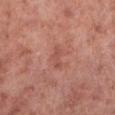biopsy status=imaged on a skin check; not biopsied
location=the left lower leg
illumination=white-light
TBP lesion metrics=a lesion color around L≈52 a*≈26 b*≈28 in CIELAB, about 7 CIELAB-L* units darker than the surrounding skin, and a normalized lesion–skin contrast near 4.5; a classifier nevus-likeness of about 0/100 and a detector confidence of about 100 out of 100 that the crop contains a lesion
lesion size=≈3 mm
subject=male, roughly 55 years of age
acquisition=total-body-photography crop, ~15 mm field of view